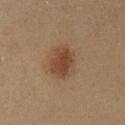Background:
Cropped from a whole-body photographic skin survey; the tile spans about 15 mm. An algorithmic analysis of the crop reported an eccentricity of roughly 0.65 and a shape-asymmetry score of about 0.15 (0 = symmetric). The analysis additionally found a border-irregularity index near 2/10 and a peripheral color-asymmetry measure near 1. And it measured a nevus-likeness score of about 100/100 and a lesion-detection confidence of about 100/100. The lesion is on the left lower leg. A female patient, aged around 50. The tile uses cross-polarized illumination. About 3.5 mm across.